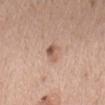Case summary:
- notes — total-body-photography surveillance lesion; no biopsy
- body site — the mid back
- image — total-body-photography crop, ~15 mm field of view
- subject — female, aged 58–62